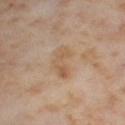Part of a total-body skin-imaging series; this lesion was reviewed on a skin check and was not flagged for biopsy. A 15 mm crop from a total-body photograph taken for skin-cancer surveillance. An algorithmic analysis of the crop reported a border-irregularity rating of about 3.5/10, a within-lesion color-variation index near 4/10, and peripheral color asymmetry of about 1.5. The lesion is located on the left thigh. Captured under cross-polarized illumination. About 5 mm across. A female patient about 55 years old.